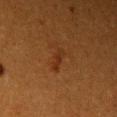Imaged during a routine full-body skin examination; the lesion was not biopsied and no histopathology is available. Automated tile analysis of the lesion measured a lesion color around L≈26 a*≈20 b*≈30 in CIELAB, about 6 CIELAB-L* units darker than the surrounding skin, and a normalized lesion–skin contrast near 6. The software also gave border irregularity of about 5 on a 0–10 scale and radial color variation of about 0. On the right upper arm. A 15 mm close-up extracted from a 3D total-body photography capture. Longest diameter approximately 3 mm. Captured under cross-polarized illumination. A female patient aged 53 to 57.